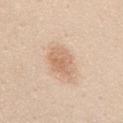notes: imaged on a skin check; not biopsied
subject: female, in their 40s
acquisition: total-body-photography crop, ~15 mm field of view
illumination: white-light
body site: the mid back